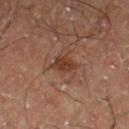The lesion was tiled from a total-body skin photograph and was not biopsied. On the left thigh. Longest diameter approximately 2.5 mm. The tile uses cross-polarized illumination. This image is a 15 mm lesion crop taken from a total-body photograph. A male subject, aged approximately 60.